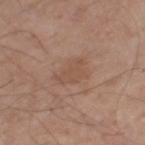  biopsy_status: not biopsied; imaged during a skin examination
  image:
    source: total-body photography crop
    field_of_view_mm: 15
  site: leg
  automated_metrics:
    area_mm2_approx: 7.0
    eccentricity: 0.75
    shape_asymmetry: 0.35
    border_irregularity_0_10: 4.0
    color_variation_0_10: 1.5
    peripheral_color_asymmetry: 0.5
  patient:
    sex: male
    age_approx: 60
  lesion_size:
    long_diameter_mm_approx: 4.0